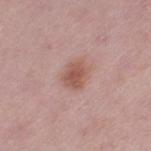Clinical impression: Imaged during a routine full-body skin examination; the lesion was not biopsied and no histopathology is available. Image and clinical context: The lesion is located on the left thigh. Imaged with white-light lighting. The subject is a female approximately 40 years of age. This image is a 15 mm lesion crop taken from a total-body photograph.